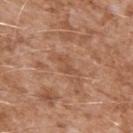No biopsy was performed on this lesion — it was imaged during a full skin examination and was not determined to be concerning. Located on the left upper arm. This is a white-light tile. Cropped from a whole-body photographic skin survey; the tile spans about 15 mm. A male subject in their mid-40s.